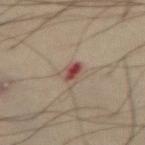workup: catalogued during a skin exam; not biopsied
location: the abdomen
TBP lesion metrics: a lesion area of about 4 mm² and an outline eccentricity of about 0.75 (0 = round, 1 = elongated)
subject: male, approximately 50 years of age
image source: 15 mm crop, total-body photography
illumination: cross-polarized